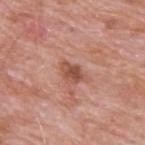site=the upper back
patient=male, roughly 60 years of age
image source=~15 mm crop, total-body skin-cancer survey
image-analysis metrics=a classifier nevus-likeness of about 0/100 and lesion-presence confidence of about 100/100
tile lighting=white-light